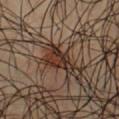The lesion was tiled from a total-body skin photograph and was not biopsied. The recorded lesion diameter is about 3.5 mm. The total-body-photography lesion software estimated a lesion area of about 7.5 mm² and an outline eccentricity of about 0.7 (0 = round, 1 = elongated). It also reported an average lesion color of about L≈29 a*≈16 b*≈23 (CIELAB) and roughly 11 lightness units darker than nearby skin. The software also gave a classifier nevus-likeness of about 5/100 and a lesion-detection confidence of about 80/100. Imaged with cross-polarized lighting. Cropped from a whole-body photographic skin survey; the tile spans about 15 mm. A male patient aged 48–52. Located on the chest.